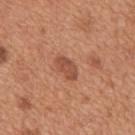The lesion was tiled from a total-body skin photograph and was not biopsied.
On the mid back.
The patient is a male approximately 65 years of age.
A 15 mm close-up extracted from a 3D total-body photography capture.